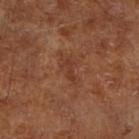Q: Automated lesion metrics?
A: an area of roughly 2.5 mm², an outline eccentricity of about 0.9 (0 = round, 1 = elongated), and a shape-asymmetry score of about 0.3 (0 = symmetric); a border-irregularity index near 3.5/10 and a within-lesion color-variation index near 0/10
Q: What kind of image is this?
A: ~15 mm crop, total-body skin-cancer survey
Q: Lesion size?
A: ≈3 mm
Q: Lesion location?
A: the right lower leg
Q: How was the tile lit?
A: cross-polarized
Q: What are the patient's age and sex?
A: aged approximately 65